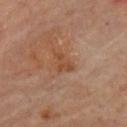Assessment: No biopsy was performed on this lesion — it was imaged during a full skin examination and was not determined to be concerning. Image and clinical context: The lesion is located on the chest. Cropped from a total-body skin-imaging series; the visible field is about 15 mm. A male patient, in their mid- to late 60s. Measured at roughly 2.5 mm in maximum diameter. The tile uses cross-polarized illumination.